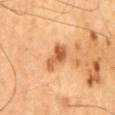Impression:
Captured during whole-body skin photography for melanoma surveillance; the lesion was not biopsied.
Image and clinical context:
A close-up tile cropped from a whole-body skin photograph, about 15 mm across. The lesion is on the mid back. An algorithmic analysis of the crop reported a footprint of about 6 mm², an eccentricity of roughly 0.85, and a shape-asymmetry score of about 0.3 (0 = symmetric). The software also gave a lesion color around L≈60 a*≈28 b*≈43 in CIELAB, about 14 CIELAB-L* units darker than the surrounding skin, and a normalized lesion–skin contrast near 8.5. The software also gave a nevus-likeness score of about 65/100 and a lesion-detection confidence of about 100/100. A male subject about 65 years old. This is a cross-polarized tile. Measured at roughly 4 mm in maximum diameter.